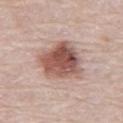Q: Was this lesion biopsied?
A: imaged on a skin check; not biopsied
Q: How was the tile lit?
A: white-light
Q: Patient demographics?
A: male, in their 80s
Q: What is the imaging modality?
A: ~15 mm crop, total-body skin-cancer survey
Q: Lesion location?
A: the abdomen